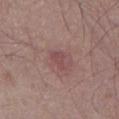{"biopsy_status": "not biopsied; imaged during a skin examination", "site": "abdomen", "lighting": "white-light", "patient": {"sex": "male", "age_approx": 75}, "automated_metrics": {"area_mm2_approx": 3.0, "eccentricity": 0.85, "shape_asymmetry": 0.5, "cielab_L": 47, "cielab_a": 23, "cielab_b": 19, "vs_skin_darker_L": 7.0, "vs_skin_contrast_norm": 5.5, "nevus_likeness_0_100": 0}, "image": {"source": "total-body photography crop", "field_of_view_mm": 15}}